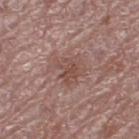Impression: The lesion was photographed on a routine skin check and not biopsied; there is no pathology result. Clinical summary: A roughly 15 mm field-of-view crop from a total-body skin photograph. From the left thigh. This is a white-light tile. The subject is a female aged 63 to 67.